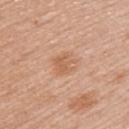Case summary:
• biopsy status: catalogued during a skin exam; not biopsied
• subject: female, aged approximately 50
• anatomic site: the left upper arm
• lesion diameter: about 2.5 mm
• tile lighting: white-light
• image source: ~15 mm crop, total-body skin-cancer survey